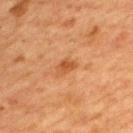Impression:
Imaged during a routine full-body skin examination; the lesion was not biopsied and no histopathology is available.
Acquisition and patient details:
The patient is a female aged 38–42. About 2.5 mm across. A region of skin cropped from a whole-body photographic capture, roughly 15 mm wide. The lesion is located on the mid back. Automated image analysis of the tile measured an average lesion color of about L≈47 a*≈24 b*≈38 (CIELAB), roughly 9 lightness units darker than nearby skin, and a normalized border contrast of about 7. The software also gave radial color variation of about 0.5.